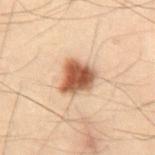biopsy status — no biopsy performed (imaged during a skin exam)
image source — 15 mm crop, total-body photography
subject — male, about 30 years old
TBP lesion metrics — an average lesion color of about L≈46 a*≈19 b*≈29 (CIELAB), a lesion–skin lightness drop of about 16, and a normalized border contrast of about 11.5; a color-variation rating of about 5/10 and peripheral color asymmetry of about 1.5; a nevus-likeness score of about 100/100 and lesion-presence confidence of about 100/100
location — the lower back
size — about 4.5 mm